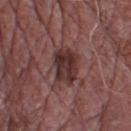Captured during whole-body skin photography for melanoma surveillance; the lesion was not biopsied. Longest diameter approximately 4.5 mm. Located on the chest. A 15 mm close-up tile from a total-body photography series done for melanoma screening. Captured under white-light illumination. A male subject, aged 68 to 72.